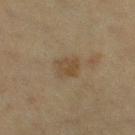Assessment:
No biopsy was performed on this lesion — it was imaged during a full skin examination and was not determined to be concerning.
Context:
A female subject, aged 48 to 52. A lesion tile, about 15 mm wide, cut from a 3D total-body photograph. Measured at roughly 2.5 mm in maximum diameter. On the leg. The lesion-visualizer software estimated a lesion area of about 5.5 mm², a shape eccentricity near 0.35, and two-axis asymmetry of about 0.3. It also reported a border-irregularity rating of about 3/10. It also reported a classifier nevus-likeness of about 0/100 and a detector confidence of about 100 out of 100 that the crop contains a lesion. The tile uses cross-polarized illumination.